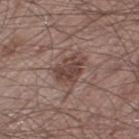Case summary:
• follow-up — catalogued during a skin exam; not biopsied
• subject — male, in their mid-60s
• image — ~15 mm crop, total-body skin-cancer survey
• site — the right thigh